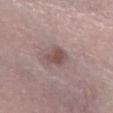Impression:
Imaged during a routine full-body skin examination; the lesion was not biopsied and no histopathology is available.
Acquisition and patient details:
This image is a 15 mm lesion crop taken from a total-body photograph. A male subject aged approximately 30.